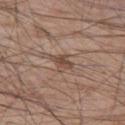A close-up tile cropped from a whole-body skin photograph, about 15 mm across. This is a white-light tile. A male patient, aged around 55. The lesion is on the left thigh.